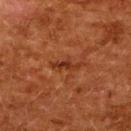Imaged during a routine full-body skin examination; the lesion was not biopsied and no histopathology is available.
This is a cross-polarized tile.
Longest diameter approximately 3.5 mm.
The total-body-photography lesion software estimated a lesion area of about 3 mm² and a symmetry-axis asymmetry near 0.35. The software also gave an average lesion color of about L≈28 a*≈24 b*≈30 (CIELAB), about 7 CIELAB-L* units darker than the surrounding skin, and a normalized lesion–skin contrast near 7.5. It also reported a border-irregularity rating of about 5/10 and radial color variation of about 0. The analysis additionally found a nevus-likeness score of about 0/100 and a detector confidence of about 100 out of 100 that the crop contains a lesion.
A female subject aged around 50.
On the upper back.
A close-up tile cropped from a whole-body skin photograph, about 15 mm across.